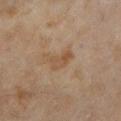The lesion was tiled from a total-body skin photograph and was not biopsied. Located on the left lower leg. A region of skin cropped from a whole-body photographic capture, roughly 15 mm wide. A female patient, approximately 60 years of age. Imaged with cross-polarized lighting.